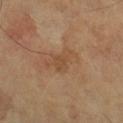A male subject aged 63–67. The tile uses cross-polarized illumination. The lesion is located on the right lower leg. Automated image analysis of the tile measured an eccentricity of roughly 0.7. The software also gave a nevus-likeness score of about 0/100 and lesion-presence confidence of about 100/100. A lesion tile, about 15 mm wide, cut from a 3D total-body photograph.